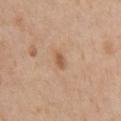{"biopsy_status": "not biopsied; imaged during a skin examination", "patient": {"sex": "male", "age_approx": 60}, "lighting": "white-light", "image": {"source": "total-body photography crop", "field_of_view_mm": 15}, "lesion_size": {"long_diameter_mm_approx": 2.5}, "site": "chest"}